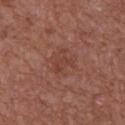Clinical impression:
Part of a total-body skin-imaging series; this lesion was reviewed on a skin check and was not flagged for biopsy.
Acquisition and patient details:
A lesion tile, about 15 mm wide, cut from a 3D total-body photograph. The lesion is located on the chest. A male subject approximately 65 years of age.